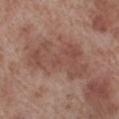Assessment:
Imaged during a routine full-body skin examination; the lesion was not biopsied and no histopathology is available.
Background:
About 8.5 mm across. Cropped from a total-body skin-imaging series; the visible field is about 15 mm. A male subject, aged approximately 70. Captured under white-light illumination. From the leg.